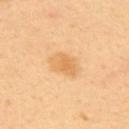Captured during whole-body skin photography for melanoma surveillance; the lesion was not biopsied. The total-body-photography lesion software estimated a lesion color around L≈70 a*≈21 b*≈45 in CIELAB and a normalized border contrast of about 6. The lesion is located on the back. Imaged with cross-polarized lighting. The recorded lesion diameter is about 3.5 mm. A male subject, aged 38 to 42. Cropped from a total-body skin-imaging series; the visible field is about 15 mm.